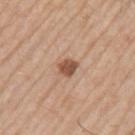automated lesion analysis = an area of roughly 4 mm² and a shape eccentricity near 0.65; an automated nevus-likeness rating near 90 out of 100 and a lesion-detection confidence of about 100/100 | image = total-body-photography crop, ~15 mm field of view | patient = male, in their mid- to late 70s | diameter = ≈2.5 mm | site = the left upper arm.